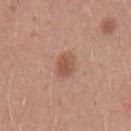Assessment: Part of a total-body skin-imaging series; this lesion was reviewed on a skin check and was not flagged for biopsy. Background: Located on the left upper arm. A male subject, about 25 years old. A lesion tile, about 15 mm wide, cut from a 3D total-body photograph. The tile uses white-light illumination.